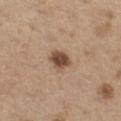Q: What is the anatomic site?
A: the left thigh
Q: What did automated image analysis measure?
A: an eccentricity of roughly 0.45 and a shape-asymmetry score of about 0.2 (0 = symmetric); a within-lesion color-variation index near 2.5/10 and radial color variation of about 1; an automated nevus-likeness rating near 95 out of 100 and lesion-presence confidence of about 100/100
Q: What lighting was used for the tile?
A: white-light illumination
Q: How large is the lesion?
A: ≈2.5 mm
Q: What kind of image is this?
A: ~15 mm crop, total-body skin-cancer survey
Q: Who is the patient?
A: male, approximately 70 years of age